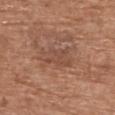Case summary:
• follow-up: catalogued during a skin exam; not biopsied
• anatomic site: the upper back
• lighting: white-light
• acquisition: 15 mm crop, total-body photography
• subject: male, aged around 70
• automated lesion analysis: a lesion color around L≈48 a*≈21 b*≈29 in CIELAB and a lesion–skin lightness drop of about 6; a nevus-likeness score of about 0/100 and a detector confidence of about 100 out of 100 that the crop contains a lesion
• diameter: ≈4.5 mm